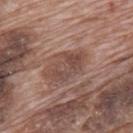notes=total-body-photography surveillance lesion; no biopsy
image=~15 mm crop, total-body skin-cancer survey
anatomic site=the back
patient=male, roughly 70 years of age
lesion size=~5 mm (longest diameter)
lighting=white-light illumination